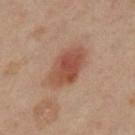Clinical impression: The lesion was photographed on a routine skin check and not biopsied; there is no pathology result. Image and clinical context: A close-up tile cropped from a whole-body skin photograph, about 15 mm across. The lesion's longest dimension is about 5 mm. On the left leg. The patient is a female aged around 40. This is a cross-polarized tile.